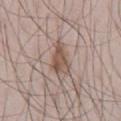<record>
<lesion_size>
  <long_diameter_mm_approx>4.5</long_diameter_mm_approx>
</lesion_size>
<patient>
  <sex>male</sex>
  <age_approx>45</age_approx>
</patient>
<site>abdomen</site>
<image>
  <source>total-body photography crop</source>
  <field_of_view_mm>15</field_of_view_mm>
</image>
<lighting>white-light</lighting>
</record>